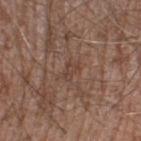notes — no biopsy performed (imaged during a skin exam); automated metrics — border irregularity of about 4 on a 0–10 scale and peripheral color asymmetry of about 0; location — the leg; lesion diameter — ~2.5 mm (longest diameter); image source — ~15 mm crop, total-body skin-cancer survey; patient — male, aged approximately 60.